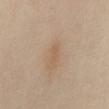workup: total-body-photography surveillance lesion; no biopsy
site: the abdomen
lighting: cross-polarized illumination
diameter: about 3 mm
subject: female, aged approximately 55
acquisition: ~15 mm tile from a whole-body skin photo